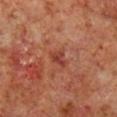Clinical impression:
Imaged during a routine full-body skin examination; the lesion was not biopsied and no histopathology is available.